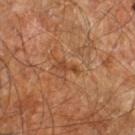Q: Was this lesion biopsied?
A: total-body-photography surveillance lesion; no biopsy
Q: Illumination type?
A: cross-polarized
Q: What did automated image analysis measure?
A: a footprint of about 2.5 mm², a shape eccentricity near 0.95, and a shape-asymmetry score of about 0.45 (0 = symmetric); a mean CIELAB color near L≈42 a*≈24 b*≈35 and a normalized lesion–skin contrast near 6.5; a border-irregularity rating of about 5.5/10, a within-lesion color-variation index near 0/10, and radial color variation of about 0; a nevus-likeness score of about 0/100
Q: Where on the body is the lesion?
A: the right leg
Q: What are the patient's age and sex?
A: male, in their 60s
Q: Lesion size?
A: ≈2.5 mm
Q: What is the imaging modality?
A: 15 mm crop, total-body photography